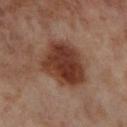Imaged during a routine full-body skin examination; the lesion was not biopsied and no histopathology is available.
Approximately 6 mm at its widest.
A female subject aged around 55.
This image is a 15 mm lesion crop taken from a total-body photograph.
The lesion is located on the right lower leg.